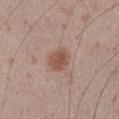Imaged during a routine full-body skin examination; the lesion was not biopsied and no histopathology is available.